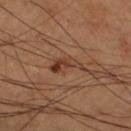follow-up=no biopsy performed (imaged during a skin exam)
image source=15 mm crop, total-body photography
anatomic site=the left lower leg
tile lighting=cross-polarized illumination
subject=male, aged 58 to 62